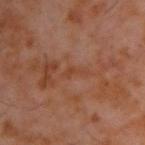Recorded during total-body skin imaging; not selected for excision or biopsy.
A 15 mm crop from a total-body photograph taken for skin-cancer surveillance.
The patient is a male aged 58–62.
Located on the upper back.
The recorded lesion diameter is about 2.5 mm.